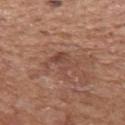This lesion was catalogued during total-body skin photography and was not selected for biopsy.
From the mid back.
This image is a 15 mm lesion crop taken from a total-body photograph.
Captured under white-light illumination.
A male patient approximately 65 years of age.
Automated image analysis of the tile measured a lesion area of about 6 mm² and a symmetry-axis asymmetry near 0.6. And it measured a lesion color around L≈46 a*≈22 b*≈27 in CIELAB, about 7 CIELAB-L* units darker than the surrounding skin, and a normalized lesion–skin contrast near 5.5. It also reported a classifier nevus-likeness of about 0/100 and a detector confidence of about 95 out of 100 that the crop contains a lesion.